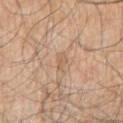The lesion was photographed on a routine skin check and not biopsied; there is no pathology result.
A male subject aged around 80.
The lesion is on the right upper arm.
Imaged with white-light lighting.
A 15 mm close-up tile from a total-body photography series done for melanoma screening.
The total-body-photography lesion software estimated a border-irregularity rating of about 3/10, a color-variation rating of about 0/10, and peripheral color asymmetry of about 0. And it measured an automated nevus-likeness rating near 0 out of 100.